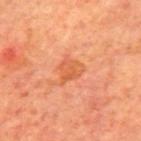{
  "biopsy_status": "not biopsied; imaged during a skin examination",
  "image": {
    "source": "total-body photography crop",
    "field_of_view_mm": 15
  },
  "lighting": "cross-polarized",
  "patient": {
    "sex": "male",
    "age_approx": 70
  },
  "lesion_size": {
    "long_diameter_mm_approx": 3.0
  },
  "automated_metrics": {
    "lesion_detection_confidence_0_100": 100
  },
  "site": "back"
}